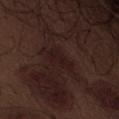biopsy_status: not biopsied; imaged during a skin examination
image:
  source: total-body photography crop
  field_of_view_mm: 15
site: abdomen
patient:
  sex: male
  age_approx: 70